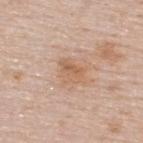– workup · catalogued during a skin exam; not biopsied
– patient · female, about 50 years old
– TBP lesion metrics · a lesion color around L≈61 a*≈19 b*≈32 in CIELAB and roughly 7 lightness units darker than nearby skin; a border-irregularity index near 3.5/10 and a within-lesion color-variation index near 4.5/10
– lesion diameter · about 3 mm
– anatomic site · the upper back
– image · total-body-photography crop, ~15 mm field of view
– lighting · white-light illumination